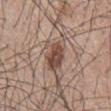Q: Was this lesion biopsied?
A: imaged on a skin check; not biopsied
Q: Where on the body is the lesion?
A: the mid back
Q: Who is the patient?
A: male, about 45 years old
Q: What did automated image analysis measure?
A: a footprint of about 9 mm², an outline eccentricity of about 0.8 (0 = round, 1 = elongated), and a symmetry-axis asymmetry near 0.35; a border-irregularity index near 4.5/10 and a within-lesion color-variation index near 4.5/10; a classifier nevus-likeness of about 95/100 and a lesion-detection confidence of about 100/100
Q: How was this image acquired?
A: total-body-photography crop, ~15 mm field of view
Q: How was the tile lit?
A: white-light illumination